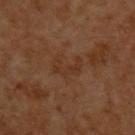The patient is a male in their 60s.
The tile uses cross-polarized illumination.
The lesion-visualizer software estimated a footprint of about 6 mm², a shape eccentricity near 0.8, and a symmetry-axis asymmetry near 0.5. It also reported an automated nevus-likeness rating near 0 out of 100.
Approximately 3.5 mm at its widest.
A 15 mm close-up tile from a total-body photography series done for melanoma screening.
The lesion is on the upper back.